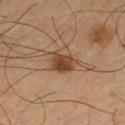biopsy_status: not biopsied; imaged during a skin examination
automated_metrics:
  area_mm2_approx: 7.0
  eccentricity: 0.7
  shape_asymmetry: 0.25
site: leg
patient:
  sex: male
  age_approx: 65
image:
  source: total-body photography crop
  field_of_view_mm: 15